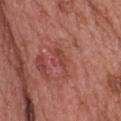{
  "biopsy_status": "not biopsied; imaged during a skin examination",
  "lesion_size": {
    "long_diameter_mm_approx": 4.0
  },
  "image": {
    "source": "total-body photography crop",
    "field_of_view_mm": 15
  },
  "patient": {
    "sex": "female",
    "age_approx": 60
  },
  "site": "upper back",
  "lighting": "white-light"
}